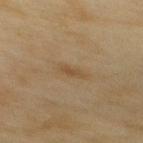* image source — ~15 mm crop, total-body skin-cancer survey
* lighting — cross-polarized
* location — the upper back
* TBP lesion metrics — an average lesion color of about L≈44 a*≈14 b*≈31 (CIELAB), about 6 CIELAB-L* units darker than the surrounding skin, and a lesion-to-skin contrast of about 5.5 (normalized; higher = more distinct); a detector confidence of about 100 out of 100 that the crop contains a lesion
* subject — female, aged approximately 60